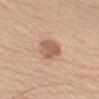<record>
<biopsy_status>not biopsied; imaged during a skin examination</biopsy_status>
<patient>
  <sex>male</sex>
  <age_approx>55</age_approx>
</patient>
<site>right upper arm</site>
<lighting>white-light</lighting>
<automated_metrics>
  <cielab_L>59</cielab_L>
  <cielab_a>22</cielab_a>
  <cielab_b>30</cielab_b>
  <vs_skin_darker_L>11.0</vs_skin_darker_L>
  <border_irregularity_0_10>1.5</border_irregularity_0_10>
  <peripheral_color_asymmetry>1.0</peripheral_color_asymmetry>
  <nevus_likeness_0_100>65</nevus_likeness_0_100>
  <lesion_detection_confidence_0_100>100</lesion_detection_confidence_0_100>
</automated_metrics>
<image>
  <source>total-body photography crop</source>
  <field_of_view_mm>15</field_of_view_mm>
</image>
</record>